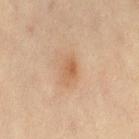Findings:
- site: the leg
- patient: female, about 45 years old
- image source: total-body-photography crop, ~15 mm field of view